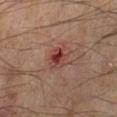Q: Illumination type?
A: cross-polarized
Q: Patient demographics?
A: male, in their mid-50s
Q: What did automated image analysis measure?
A: an automated nevus-likeness rating near 0 out of 100 and a detector confidence of about 100 out of 100 that the crop contains a lesion
Q: What is the imaging modality?
A: ~15 mm crop, total-body skin-cancer survey
Q: What is the lesion's diameter?
A: ~2.5 mm (longest diameter)
Q: Where on the body is the lesion?
A: the left lower leg